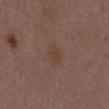Captured during whole-body skin photography for melanoma surveillance; the lesion was not biopsied. A 15 mm close-up tile from a total-body photography series done for melanoma screening. The subject is a male aged 48 to 52. The lesion is on the chest. Longest diameter approximately 2.5 mm. The tile uses white-light illumination.